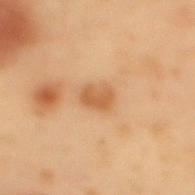No biopsy was performed on this lesion — it was imaged during a full skin examination and was not determined to be concerning. Cropped from a whole-body photographic skin survey; the tile spans about 15 mm. A male subject, in their mid- to late 50s. The lesion is on the mid back.